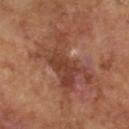notes — catalogued during a skin exam; not biopsied
diameter — about 7.5 mm
lighting — cross-polarized
TBP lesion metrics — a shape eccentricity near 0.75 and a shape-asymmetry score of about 0.35 (0 = symmetric); a lesion-detection confidence of about 100/100
patient — male, in their mid-60s
image — ~15 mm tile from a whole-body skin photo